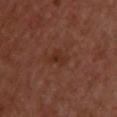Impression:
No biopsy was performed on this lesion — it was imaged during a full skin examination and was not determined to be concerning.
Clinical summary:
The total-body-photography lesion software estimated a border-irregularity rating of about 3.5/10 and peripheral color asymmetry of about 0.5. This is a cross-polarized tile. The subject is a male aged 48 to 52. A 15 mm close-up extracted from a 3D total-body photography capture. The recorded lesion diameter is about 3 mm. The lesion is located on the upper back.